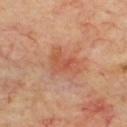notes: no biopsy performed (imaged during a skin exam) | diameter: about 3.5 mm | patient: male, about 70 years old | site: the front of the torso | image source: ~15 mm crop, total-body skin-cancer survey.